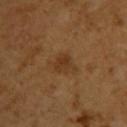Imaged during a routine full-body skin examination; the lesion was not biopsied and no histopathology is available. The patient is a female roughly 55 years of age. Longest diameter approximately 2.5 mm. A 15 mm crop from a total-body photograph taken for skin-cancer surveillance. This is a cross-polarized tile. The lesion is on the upper back.